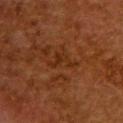{"biopsy_status": "not biopsied; imaged during a skin examination", "site": "upper back", "image": {"source": "total-body photography crop", "field_of_view_mm": 15}, "lighting": "cross-polarized", "patient": {"sex": "female", "age_approx": 50}, "lesion_size": {"long_diameter_mm_approx": 4.0}}